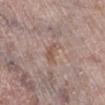biopsy status: imaged on a skin check; not biopsied | size: ~3 mm (longest diameter) | location: the right lower leg | imaging modality: ~15 mm tile from a whole-body skin photo | patient: female, in their mid- to late 60s.